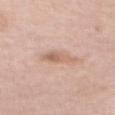  biopsy_status: not biopsied; imaged during a skin examination
  lighting: white-light
  automated_metrics:
    area_mm2_approx: 5.0
    eccentricity: 0.85
    border_irregularity_0_10: 4.0
    lesion_detection_confidence_0_100: 100
  site: abdomen
  patient:
    sex: female
    age_approx: 65
  image:
    source: total-body photography crop
    field_of_view_mm: 15